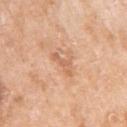Part of a total-body skin-imaging series; this lesion was reviewed on a skin check and was not flagged for biopsy.
An algorithmic analysis of the crop reported a footprint of about 3.5 mm² and an outline eccentricity of about 0.9 (0 = round, 1 = elongated). It also reported an automated nevus-likeness rating near 0 out of 100 and lesion-presence confidence of about 100/100.
About 3.5 mm across.
Cropped from a total-body skin-imaging series; the visible field is about 15 mm.
On the left upper arm.
This is a white-light tile.
A female subject, aged 73–77.